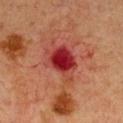Recorded during total-body skin imaging; not selected for excision or biopsy.
The subject is a female aged approximately 40.
A roughly 15 mm field-of-view crop from a total-body skin photograph.
The lesion's longest dimension is about 4.5 mm.
The lesion is on the chest.
Captured under cross-polarized illumination.
The total-body-photography lesion software estimated an average lesion color of about L≈31 a*≈34 b*≈26 (CIELAB), a lesion–skin lightness drop of about 12, and a lesion-to-skin contrast of about 10.5 (normalized; higher = more distinct). And it measured a color-variation rating of about 5.5/10 and peripheral color asymmetry of about 1.